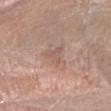Located on the right forearm.
Cropped from a total-body skin-imaging series; the visible field is about 15 mm.
The patient is a female aged approximately 60.
Imaged with white-light lighting.
The total-body-photography lesion software estimated a mean CIELAB color near L≈57 a*≈18 b*≈25 and a normalized border contrast of about 4.5. It also reported an automated nevus-likeness rating near 0 out of 100 and a detector confidence of about 100 out of 100 that the crop contains a lesion.